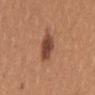<case>
<biopsy_status>not biopsied; imaged during a skin examination</biopsy_status>
<site>mid back</site>
<lighting>white-light</lighting>
<patient>
  <sex>female</sex>
  <age_approx>35</age_approx>
</patient>
<lesion_size>
  <long_diameter_mm_approx>3.5</long_diameter_mm_approx>
</lesion_size>
<image>
  <source>total-body photography crop</source>
  <field_of_view_mm>15</field_of_view_mm>
</image>
</case>